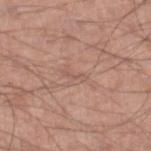{"biopsy_status": "not biopsied; imaged during a skin examination", "lesion_size": {"long_diameter_mm_approx": 3.0}, "patient": {"sex": "male", "age_approx": 60}, "image": {"source": "total-body photography crop", "field_of_view_mm": 15}, "site": "right lower leg", "lighting": "white-light"}